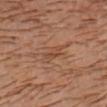Assessment: Part of a total-body skin-imaging series; this lesion was reviewed on a skin check and was not flagged for biopsy. Background: This is a cross-polarized tile. From the head or neck. Approximately 3 mm at its widest. A male subject, aged around 30. Cropped from a total-body skin-imaging series; the visible field is about 15 mm.